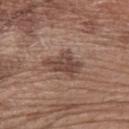automated_metrics:
  vs_skin_darker_L: 10.0
  vs_skin_contrast_norm: 8.0
  nevus_likeness_0_100: 45
  lesion_detection_confidence_0_100: 100
site: left forearm
lesion_size:
  long_diameter_mm_approx: 4.5
patient:
  sex: male
  age_approx: 70
lighting: white-light
image:
  source: total-body photography crop
  field_of_view_mm: 15